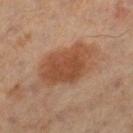Notes:
* workup: catalogued during a skin exam; not biopsied
* lesion diameter: ~7 mm (longest diameter)
* anatomic site: the left thigh
* lighting: cross-polarized
* patient: male, in their 60s
* acquisition: ~15 mm tile from a whole-body skin photo